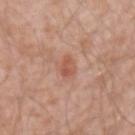Impression:
The lesion was tiled from a total-body skin photograph and was not biopsied.
Clinical summary:
Cropped from a whole-body photographic skin survey; the tile spans about 15 mm. The lesion is on the right upper arm. A male subject, in their 60s.